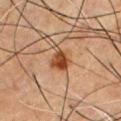Part of a total-body skin-imaging series; this lesion was reviewed on a skin check and was not flagged for biopsy.
Automated tile analysis of the lesion measured a border-irregularity index near 3/10, a within-lesion color-variation index near 4/10, and a peripheral color-asymmetry measure near 1.5.
On the chest.
A region of skin cropped from a whole-body photographic capture, roughly 15 mm wide.
A male patient, aged 48–52.
Approximately 3 mm at its widest.
Captured under cross-polarized illumination.